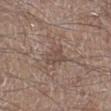Part of a total-body skin-imaging series; this lesion was reviewed on a skin check and was not flagged for biopsy.
About 3.5 mm across.
The lesion is located on the leg.
A lesion tile, about 15 mm wide, cut from a 3D total-body photograph.
A male patient aged 58 to 62.